A female patient aged 58–62 · a region of skin cropped from a whole-body photographic capture, roughly 15 mm wide · on the left upper arm — 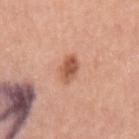Biopsy histopathology demonstrated a benign skin lesion: dysplastic (Clark) nevus.